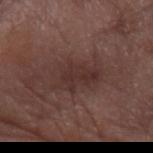* workup · catalogued during a skin exam; not biopsied
* image · ~15 mm tile from a whole-body skin photo
* diameter · ~5.5 mm (longest diameter)
* subject · male, in their mid-70s
* location · the left forearm
* tile lighting · white-light